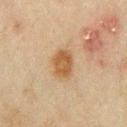Assessment:
Captured during whole-body skin photography for melanoma surveillance; the lesion was not biopsied.
Background:
A male subject, aged approximately 70. From the front of the torso. A 15 mm close-up tile from a total-body photography series done for melanoma screening. The recorded lesion diameter is about 3.5 mm. The total-body-photography lesion software estimated a mean CIELAB color near L≈46 a*≈16 b*≈33, about 9 CIELAB-L* units darker than the surrounding skin, and a lesion-to-skin contrast of about 8.5 (normalized; higher = more distinct). The software also gave a border-irregularity rating of about 1.5/10, a within-lesion color-variation index near 3/10, and peripheral color asymmetry of about 1. The software also gave a nevus-likeness score of about 95/100 and a lesion-detection confidence of about 100/100.